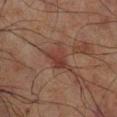Image and clinical context:
Captured under cross-polarized illumination. The subject is a male roughly 70 years of age. Measured at roughly 3.5 mm in maximum diameter. From the right lower leg. Cropped from a whole-body photographic skin survey; the tile spans about 15 mm. An algorithmic analysis of the crop reported a lesion area of about 6 mm², an eccentricity of roughly 0.7, and two-axis asymmetry of about 0.45. And it measured border irregularity of about 4.5 on a 0–10 scale, internal color variation of about 3 on a 0–10 scale, and peripheral color asymmetry of about 1. The software also gave a nevus-likeness score of about 0/100.